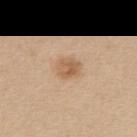A roughly 15 mm field-of-view crop from a total-body skin photograph.
Captured under white-light illumination.
A female subject approximately 45 years of age.
The lesion is located on the upper back.
Measured at roughly 2.5 mm in maximum diameter.